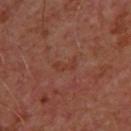This lesion was catalogued during total-body skin photography and was not selected for biopsy.
On the upper back.
A lesion tile, about 15 mm wide, cut from a 3D total-body photograph.
A male patient aged 48–52.
The tile uses cross-polarized illumination.
Automated image analysis of the tile measured a footprint of about 3.5 mm² and a symmetry-axis asymmetry near 0.35. The analysis additionally found an average lesion color of about L≈37 a*≈24 b*≈28 (CIELAB). The software also gave a border-irregularity rating of about 4/10 and internal color variation of about 1 on a 0–10 scale. And it measured an automated nevus-likeness rating near 0 out of 100.